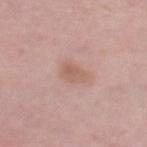Captured during whole-body skin photography for melanoma surveillance; the lesion was not biopsied.
Measured at roughly 3 mm in maximum diameter.
A region of skin cropped from a whole-body photographic capture, roughly 15 mm wide.
This is a white-light tile.
The subject is a female aged 38–42.
The lesion is located on the right thigh.
The lesion-visualizer software estimated a footprint of about 5 mm², a shape eccentricity near 0.7, and a shape-asymmetry score of about 0.25 (0 = symmetric). The analysis additionally found an average lesion color of about L≈59 a*≈20 b*≈26 (CIELAB), a lesion–skin lightness drop of about 7, and a normalized lesion–skin contrast near 5.5.